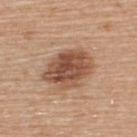{"biopsy_status": "not biopsied; imaged during a skin examination", "patient": {"sex": "male", "age_approx": 55}, "image": {"source": "total-body photography crop", "field_of_view_mm": 15}, "site": "upper back"}